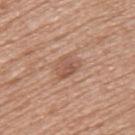follow-up: total-body-photography surveillance lesion; no biopsy
subject: female, in their 50s
anatomic site: the upper back
TBP lesion metrics: a lesion area of about 5.5 mm², an eccentricity of roughly 0.6, and a symmetry-axis asymmetry near 0.25; an average lesion color of about L≈54 a*≈21 b*≈29 (CIELAB) and a lesion-to-skin contrast of about 6 (normalized; higher = more distinct); a border-irregularity rating of about 2.5/10 and a within-lesion color-variation index near 3.5/10
image: total-body-photography crop, ~15 mm field of view
tile lighting: white-light
size: about 3 mm